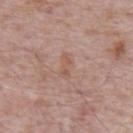Assessment: Part of a total-body skin-imaging series; this lesion was reviewed on a skin check and was not flagged for biopsy. Acquisition and patient details: A roughly 15 mm field-of-view crop from a total-body skin photograph. The patient is a male aged around 70. Located on the abdomen. The lesion's longest dimension is about 2.5 mm. Automated image analysis of the tile measured a lesion area of about 2 mm², an eccentricity of roughly 0.95, and a shape-asymmetry score of about 0.6 (0 = symmetric). It also reported about 6 CIELAB-L* units darker than the surrounding skin. The analysis additionally found border irregularity of about 7 on a 0–10 scale and peripheral color asymmetry of about 0. It also reported a nevus-likeness score of about 0/100.